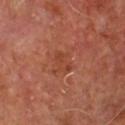– lighting · cross-polarized illumination
– lesion diameter · about 2.5 mm
– body site · the chest
– subject · male, aged around 65
– acquisition · total-body-photography crop, ~15 mm field of view